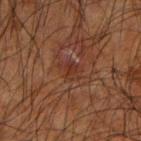Part of a total-body skin-imaging series; this lesion was reviewed on a skin check and was not flagged for biopsy. A lesion tile, about 15 mm wide, cut from a 3D total-body photograph. Located on the right upper arm. A male subject in their mid-60s. This is a cross-polarized tile. Longest diameter approximately 2.5 mm.